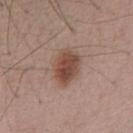No biopsy was performed on this lesion — it was imaged during a full skin examination and was not determined to be concerning. This is a white-light tile. About 4 mm across. A close-up tile cropped from a whole-body skin photograph, about 15 mm across. A male patient, in their mid- to late 50s.